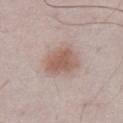Captured during whole-body skin photography for melanoma surveillance; the lesion was not biopsied. A lesion tile, about 15 mm wide, cut from a 3D total-body photograph. Longest diameter approximately 4 mm. From the front of the torso. A male subject, about 50 years old.